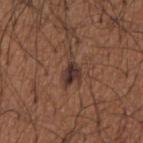{"biopsy_status": "not biopsied; imaged during a skin examination", "patient": {"sex": "male", "age_approx": 65}, "image": {"source": "total-body photography crop", "field_of_view_mm": 15}, "site": "right upper arm"}